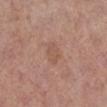workup: imaged on a skin check; not biopsied
anatomic site: the left lower leg
lesion size: about 2.5 mm
imaging modality: total-body-photography crop, ~15 mm field of view
automated lesion analysis: a mean CIELAB color near L≈53 a*≈20 b*≈28 and a normalized border contrast of about 4.5; border irregularity of about 3.5 on a 0–10 scale and a color-variation rating of about 1/10; a nevus-likeness score of about 0/100
patient: female, roughly 60 years of age
illumination: white-light illumination